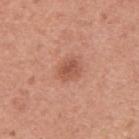No biopsy was performed on this lesion — it was imaged during a full skin examination and was not determined to be concerning. A male patient, aged 48–52. The lesion is on the back. This image is a 15 mm lesion crop taken from a total-body photograph. The recorded lesion diameter is about 2.5 mm. The lesion-visualizer software estimated a shape eccentricity near 0.6. It also reported lesion-presence confidence of about 100/100.